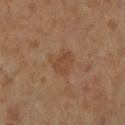Imaged during a routine full-body skin examination; the lesion was not biopsied and no histopathology is available. The lesion is located on the right lower leg. The subject is a female roughly 65 years of age. Imaged with cross-polarized lighting. A lesion tile, about 15 mm wide, cut from a 3D total-body photograph. Automated tile analysis of the lesion measured a mean CIELAB color near L≈44 a*≈19 b*≈30. And it measured border irregularity of about 3 on a 0–10 scale, a color-variation rating of about 2.5/10, and peripheral color asymmetry of about 1. The software also gave a detector confidence of about 100 out of 100 that the crop contains a lesion.